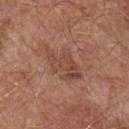Part of a total-body skin-imaging series; this lesion was reviewed on a skin check and was not flagged for biopsy.
A male patient aged 53–57.
Imaged with white-light lighting.
The lesion-visualizer software estimated a lesion area of about 11 mm², a shape eccentricity near 0.85, and a shape-asymmetry score of about 0.35 (0 = symmetric). It also reported an automated nevus-likeness rating near 0 out of 100 and lesion-presence confidence of about 100/100.
About 5.5 mm across.
From the left upper arm.
A 15 mm crop from a total-body photograph taken for skin-cancer surveillance.